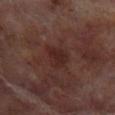<lesion>
  <biopsy_status>not biopsied; imaged during a skin examination</biopsy_status>
  <image>
    <source>total-body photography crop</source>
    <field_of_view_mm>15</field_of_view_mm>
  </image>
  <automated_metrics>
    <area_mm2_approx>6.5</area_mm2_approx>
    <eccentricity>0.6</eccentricity>
    <shape_asymmetry>0.35</shape_asymmetry>
    <border_irregularity_0_10>3.0</border_irregularity_0_10>
    <color_variation_0_10>2.0</color_variation_0_10>
    <peripheral_color_asymmetry>0.5</peripheral_color_asymmetry>
    <nevus_likeness_0_100>0</nevus_likeness_0_100>
    <lesion_detection_confidence_0_100>100</lesion_detection_confidence_0_100>
  </automated_metrics>
  <lighting>cross-polarized</lighting>
  <lesion_size>
    <long_diameter_mm_approx>3.5</long_diameter_mm_approx>
  </lesion_size>
  <patient>
    <sex>male</sex>
    <age_approx>70</age_approx>
  </patient>
  <site>leg</site>
</lesion>